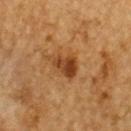The lesion was photographed on a routine skin check and not biopsied; there is no pathology result. A male subject, aged 83 or older. A 15 mm close-up tile from a total-body photography series done for melanoma screening. On the upper back.